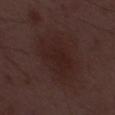Findings:
• lesion size · ~6.5 mm (longest diameter)
• illumination · white-light
• patient · male, aged 48 to 52
• imaging modality · ~15 mm crop, total-body skin-cancer survey